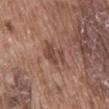Case summary:
* workup · total-body-photography surveillance lesion; no biopsy
* size · about 4 mm
* acquisition · ~15 mm tile from a whole-body skin photo
* body site · the abdomen
* patient · male, roughly 75 years of age
* tile lighting · white-light illumination